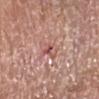Clinical impression: Captured during whole-body skin photography for melanoma surveillance; the lesion was not biopsied. Image and clinical context: A female subject, aged 73 to 77. A close-up tile cropped from a whole-body skin photograph, about 15 mm across. The lesion is located on the left lower leg.